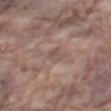<tbp_lesion>
  <biopsy_status>not biopsied; imaged during a skin examination</biopsy_status>
  <patient>
    <sex>male</sex>
    <age_approx>75</age_approx>
  </patient>
  <site>mid back</site>
  <image>
    <source>total-body photography crop</source>
    <field_of_view_mm>15</field_of_view_mm>
  </image>
</tbp_lesion>